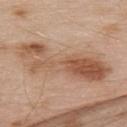| field | value |
|---|---|
| workup | total-body-photography surveillance lesion; no biopsy |
| subject | male, aged approximately 55 |
| automated metrics | a lesion area of about 34 mm² and an eccentricity of roughly 0.95; an average lesion color of about L≈56 a*≈19 b*≈31 (CIELAB) and a normalized lesion–skin contrast near 7; border irregularity of about 4.5 on a 0–10 scale, a color-variation rating of about 9/10, and peripheral color asymmetry of about 3.5; an automated nevus-likeness rating near 0 out of 100 |
| site | the upper back |
| acquisition | ~15 mm tile from a whole-body skin photo |
| size | ~11.5 mm (longest diameter) |
| lighting | white-light |